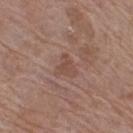Part of a total-body skin-imaging series; this lesion was reviewed on a skin check and was not flagged for biopsy. About 3 mm across. A female subject roughly 70 years of age. Cropped from a whole-body photographic skin survey; the tile spans about 15 mm. From the right thigh.